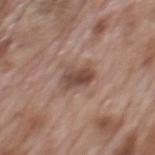{"lesion_size": {"long_diameter_mm_approx": 3.5}, "patient": {"sex": "male", "age_approx": 70}, "site": "back", "image": {"source": "total-body photography crop", "field_of_view_mm": 15}, "automated_metrics": {"border_irregularity_0_10": 2.5, "color_variation_0_10": 5.0, "peripheral_color_asymmetry": 2.0, "nevus_likeness_0_100": 85, "lesion_detection_confidence_0_100": 100}, "lighting": "white-light"}